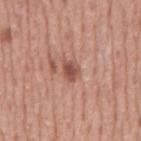Acquisition and patient details:
The lesion is located on the mid back. Measured at roughly 2.5 mm in maximum diameter. A 15 mm close-up extracted from a 3D total-body photography capture. Automated image analysis of the tile measured an area of roughly 4 mm², an outline eccentricity of about 0.7 (0 = round, 1 = elongated), and a symmetry-axis asymmetry near 0.35. The software also gave a mean CIELAB color near L≈51 a*≈24 b*≈26 and a lesion-to-skin contrast of about 8 (normalized; higher = more distinct). It also reported a classifier nevus-likeness of about 60/100 and lesion-presence confidence of about 100/100. Imaged with white-light lighting. A male subject in their mid-70s.